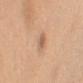{
  "biopsy_status": "not biopsied; imaged during a skin examination",
  "patient": {
    "sex": "female",
    "age_approx": 55
  },
  "image": {
    "source": "total-body photography crop",
    "field_of_view_mm": 15
  },
  "lesion_size": {
    "long_diameter_mm_approx": 3.0
  },
  "automated_metrics": {
    "area_mm2_approx": 3.0,
    "eccentricity": 0.9,
    "shape_asymmetry": 0.25,
    "border_irregularity_0_10": 3.0,
    "color_variation_0_10": 2.5,
    "peripheral_color_asymmetry": 1.0,
    "nevus_likeness_0_100": 65,
    "lesion_detection_confidence_0_100": 100
  },
  "lighting": "white-light",
  "site": "right upper arm"
}